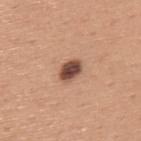The lesion was photographed on a routine skin check and not biopsied; there is no pathology result.
This is a white-light tile.
The recorded lesion diameter is about 3 mm.
This image is a 15 mm lesion crop taken from a total-body photograph.
A male patient, approximately 45 years of age.
From the upper back.
The lesion-visualizer software estimated border irregularity of about 1 on a 0–10 scale, internal color variation of about 3.5 on a 0–10 scale, and radial color variation of about 1.